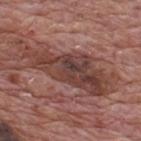No biopsy was performed on this lesion — it was imaged during a full skin examination and was not determined to be concerning.
About 10 mm across.
A male subject approximately 70 years of age.
From the back.
This image is a 15 mm lesion crop taken from a total-body photograph.